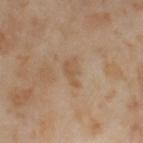The lesion was photographed on a routine skin check and not biopsied; there is no pathology result.
A 15 mm close-up extracted from a 3D total-body photography capture.
About 3.5 mm across.
The subject is a female aged 53 to 57.
The lesion is located on the left thigh.
The lesion-visualizer software estimated internal color variation of about 2 on a 0–10 scale and radial color variation of about 0.5. It also reported an automated nevus-likeness rating near 0 out of 100 and a detector confidence of about 100 out of 100 that the crop contains a lesion.